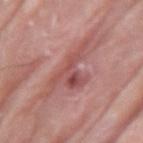Clinical impression: This lesion was catalogued during total-body skin photography and was not selected for biopsy. Image and clinical context: The lesion is located on the arm. A male patient, aged 83 or over. Cropped from a whole-body photographic skin survey; the tile spans about 15 mm.